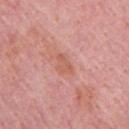{"biopsy_status": "not biopsied; imaged during a skin examination", "automated_metrics": {"border_irregularity_0_10": 3.5, "nevus_likeness_0_100": 0, "lesion_detection_confidence_0_100": 100}, "image": {"source": "total-body photography crop", "field_of_view_mm": 15}, "lesion_size": {"long_diameter_mm_approx": 3.0}, "patient": {"sex": "male", "age_approx": 65}, "site": "upper back"}